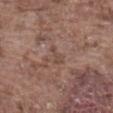This lesion was catalogued during total-body skin photography and was not selected for biopsy.